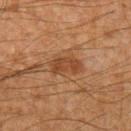No biopsy was performed on this lesion — it was imaged during a full skin examination and was not determined to be concerning. A roughly 15 mm field-of-view crop from a total-body skin photograph. The total-body-photography lesion software estimated a footprint of about 6 mm² and a shape-asymmetry score of about 0.25 (0 = symmetric). The software also gave a lesion–skin lightness drop of about 8 and a normalized lesion–skin contrast near 7. The analysis additionally found a classifier nevus-likeness of about 45/100 and lesion-presence confidence of about 100/100. The lesion is located on the left upper arm. A male subject aged approximately 50.